Located on the upper back; the subject is a female roughly 65 years of age; the lesion's longest dimension is about 4 mm; a region of skin cropped from a whole-body photographic capture, roughly 15 mm wide — 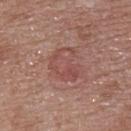Histopathology of the biopsied lesion showed a benign skin lesion: congenital melanocytic nevus.